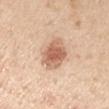Recorded during total-body skin imaging; not selected for excision or biopsy. A 15 mm crop from a total-body photograph taken for skin-cancer surveillance. On the right upper arm. Imaged with white-light lighting. The subject is a male roughly 75 years of age. Approximately 4.5 mm at its widest. The lesion-visualizer software estimated internal color variation of about 4 on a 0–10 scale and a peripheral color-asymmetry measure near 1.